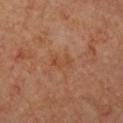Clinical impression:
Recorded during total-body skin imaging; not selected for excision or biopsy.
Clinical summary:
A female patient, aged approximately 40. The lesion is on the chest. Cropped from a total-body skin-imaging series; the visible field is about 15 mm.